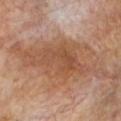Acquisition and patient details:
The patient is a male aged 68–72. The lesion-visualizer software estimated a footprint of about 16 mm², an eccentricity of roughly 0.9, and two-axis asymmetry of about 0.4. It also reported a normalized border contrast of about 7. The software also gave internal color variation of about 3.5 on a 0–10 scale and radial color variation of about 1. The analysis additionally found a classifier nevus-likeness of about 0/100. This image is a 15 mm lesion crop taken from a total-body photograph. The tile uses cross-polarized illumination. On the left lower leg. The recorded lesion diameter is about 7.5 mm.